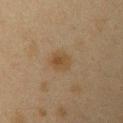image:
  source: total-body photography crop
  field_of_view_mm: 15
automated_metrics:
  border_irregularity_0_10: 1.5
  peripheral_color_asymmetry: 1.0
  lesion_detection_confidence_0_100: 100
lesion_size:
  long_diameter_mm_approx: 2.5
lighting: cross-polarized
site: left upper arm
patient:
  sex: female
  age_approx: 40